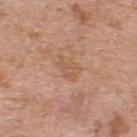Captured during whole-body skin photography for melanoma surveillance; the lesion was not biopsied. A 15 mm close-up extracted from a 3D total-body photography capture. Captured under white-light illumination. A male patient aged 53–57. Measured at roughly 3 mm in maximum diameter. Located on the upper back.